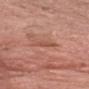Assessment: Part of a total-body skin-imaging series; this lesion was reviewed on a skin check and was not flagged for biopsy. Background: A male subject aged 78–82. The lesion is on the head or neck. The tile uses white-light illumination. Cropped from a total-body skin-imaging series; the visible field is about 15 mm. The lesion's longest dimension is about 3 mm.